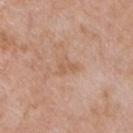Impression: The lesion was photographed on a routine skin check and not biopsied; there is no pathology result. Clinical summary: From the chest. A 15 mm close-up extracted from a 3D total-body photography capture. This is a white-light tile. Approximately 2.5 mm at its widest. The total-body-photography lesion software estimated an area of roughly 3 mm², an outline eccentricity of about 0.8 (0 = round, 1 = elongated), and a shape-asymmetry score of about 0.45 (0 = symmetric). The analysis additionally found about 6 CIELAB-L* units darker than the surrounding skin and a lesion-to-skin contrast of about 5 (normalized; higher = more distinct). The analysis additionally found a color-variation rating of about 1/10 and a peripheral color-asymmetry measure near 0.5. The software also gave an automated nevus-likeness rating near 0 out of 100. The subject is a male aged approximately 50.